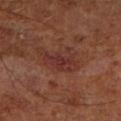Impression: No biopsy was performed on this lesion — it was imaged during a full skin examination and was not determined to be concerning. Background: Cropped from a whole-body photographic skin survey; the tile spans about 15 mm. Automated tile analysis of the lesion measured an area of roughly 10 mm², a shape eccentricity near 0.8, and two-axis asymmetry of about 0.45. And it measured a border-irregularity index near 6.5/10, a within-lesion color-variation index near 3/10, and radial color variation of about 1. Located on the right lower leg. Imaged with cross-polarized lighting. The patient is a male aged 68–72.